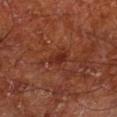Clinical impression: This lesion was catalogued during total-body skin photography and was not selected for biopsy. Background: A male patient, roughly 65 years of age. From the right lower leg. Longest diameter approximately 2.5 mm. A roughly 15 mm field-of-view crop from a total-body skin photograph. The total-body-photography lesion software estimated an area of roughly 4 mm² and a shape eccentricity near 0.7. It also reported border irregularity of about 2.5 on a 0–10 scale, a within-lesion color-variation index near 2.5/10, and peripheral color asymmetry of about 1. The tile uses cross-polarized illumination.